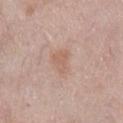biopsy status — no biopsy performed (imaged during a skin exam) | subject — female, aged 63–67 | lesion diameter — ≈3 mm | body site — the right lower leg | automated lesion analysis — a footprint of about 5 mm², a shape eccentricity near 0.55, and a shape-asymmetry score of about 0.4 (0 = symmetric); an automated nevus-likeness rating near 0 out of 100 and lesion-presence confidence of about 100/100 | tile lighting — white-light illumination | image source — total-body-photography crop, ~15 mm field of view.